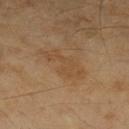workup: imaged on a skin check; not biopsied
image source: ~15 mm tile from a whole-body skin photo
tile lighting: cross-polarized illumination
size: ~6 mm (longest diameter)
location: the arm
patient: female, roughly 60 years of age